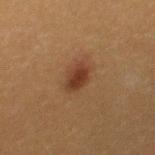Imaged during a routine full-body skin examination; the lesion was not biopsied and no histopathology is available. On the right thigh. The subject is a female aged 38–42. A 15 mm close-up extracted from a 3D total-body photography capture. About 3 mm across. Imaged with cross-polarized lighting.